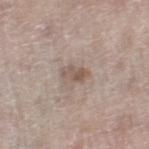{"biopsy_status": "not biopsied; imaged during a skin examination", "site": "leg", "patient": {"sex": "female", "age_approx": 60}, "image": {"source": "total-body photography crop", "field_of_view_mm": 15}}